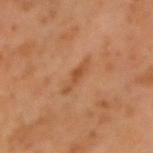workup — total-body-photography surveillance lesion; no biopsy
patient — male, roughly 60 years of age
diameter — about 3.5 mm
image source — total-body-photography crop, ~15 mm field of view
site — the right upper arm
illumination — cross-polarized
TBP lesion metrics — an average lesion color of about L≈47 a*≈23 b*≈35 (CIELAB) and a lesion–skin lightness drop of about 7; a classifier nevus-likeness of about 0/100